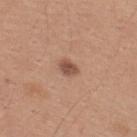Recorded during total-body skin imaging; not selected for excision or biopsy. The lesion's longest dimension is about 2.5 mm. A 15 mm crop from a total-body photograph taken for skin-cancer surveillance. A male subject, aged approximately 30. The lesion is located on the upper back. Automated image analysis of the tile measured an average lesion color of about L≈51 a*≈21 b*≈29 (CIELAB), a lesion–skin lightness drop of about 12, and a normalized border contrast of about 8.5. The analysis additionally found a border-irregularity index near 2/10, a within-lesion color-variation index near 2/10, and peripheral color asymmetry of about 0.5. The tile uses white-light illumination.